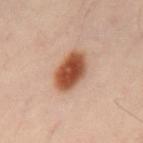From the back. The recorded lesion diameter is about 5 mm. A region of skin cropped from a whole-body photographic capture, roughly 15 mm wide. A male subject, roughly 45 years of age. Imaged with cross-polarized lighting. The total-body-photography lesion software estimated a mean CIELAB color near L≈50 a*≈25 b*≈33, a lesion–skin lightness drop of about 18, and a normalized lesion–skin contrast near 12.5.